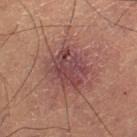Assessment:
Recorded during total-body skin imaging; not selected for excision or biopsy.
Background:
The total-body-photography lesion software estimated two-axis asymmetry of about 0.2. The analysis additionally found a lesion color around L≈37 a*≈21 b*≈17 in CIELAB, a lesion–skin lightness drop of about 8, and a normalized border contrast of about 8. And it measured a border-irregularity rating of about 2.5/10, internal color variation of about 4.5 on a 0–10 scale, and peripheral color asymmetry of about 1.5. Measured at roughly 4.5 mm in maximum diameter. The patient is a male roughly 60 years of age. A close-up tile cropped from a whole-body skin photograph, about 15 mm across. The tile uses cross-polarized illumination.